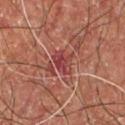<record>
<biopsy_status>not biopsied; imaged during a skin examination</biopsy_status>
<patient>
  <sex>male</sex>
  <age_approx>55</age_approx>
</patient>
<image>
  <source>total-body photography crop</source>
  <field_of_view_mm>15</field_of_view_mm>
</image>
<automated_metrics>
  <cielab_L>37</cielab_L>
  <cielab_a>28</cielab_a>
  <cielab_b>22</cielab_b>
  <vs_skin_contrast_norm>7.0</vs_skin_contrast_norm>
  <nevus_likeness_0_100>0</nevus_likeness_0_100>
  <lesion_detection_confidence_0_100>65</lesion_detection_confidence_0_100>
</automated_metrics>
<lesion_size>
  <long_diameter_mm_approx>3.0</long_diameter_mm_approx>
</lesion_size>
<site>chest</site>
<lighting>cross-polarized</lighting>
</record>